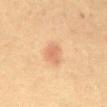Case summary:
• follow-up: catalogued during a skin exam; not biopsied
• subject: female, roughly 55 years of age
• tile lighting: cross-polarized
• imaging modality: ~15 mm tile from a whole-body skin photo
• anatomic site: the front of the torso